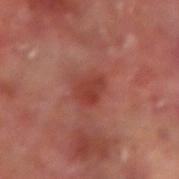Clinical impression: No biopsy was performed on this lesion — it was imaged during a full skin examination and was not determined to be concerning. Background: A male subject, roughly 65 years of age. A 15 mm close-up tile from a total-body photography series done for melanoma screening. Longest diameter approximately 3 mm. The lesion is on the left forearm. Imaged with cross-polarized lighting.